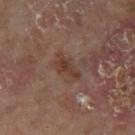follow-up=no biopsy performed (imaged during a skin exam)
automated metrics=an average lesion color of about L≈37 a*≈18 b*≈25 (CIELAB) and about 7 CIELAB-L* units darker than the surrounding skin; an automated nevus-likeness rating near 15 out of 100
subject=female, about 60 years old
anatomic site=the left lower leg
size=≈3.5 mm
imaging modality=~15 mm tile from a whole-body skin photo